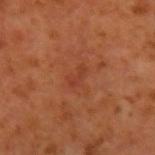Imaged during a routine full-body skin examination; the lesion was not biopsied and no histopathology is available.